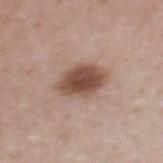workup: imaged on a skin check; not biopsied | acquisition: ~15 mm crop, total-body skin-cancer survey | subject: female, aged 38–42 | site: the upper back.